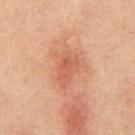Impression:
No biopsy was performed on this lesion — it was imaged during a full skin examination and was not determined to be concerning.
Acquisition and patient details:
This image is a 15 mm lesion crop taken from a total-body photograph. The patient is a male in their mid- to late 60s. From the mid back.